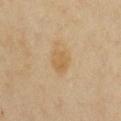Notes:
– notes — no biopsy performed (imaged during a skin exam)